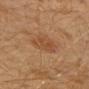biopsy_status: not biopsied; imaged during a skin examination
image:
  source: total-body photography crop
  field_of_view_mm: 15
site: left arm
lighting: cross-polarized
patient:
  sex: male
  age_approx: 30
lesion_size:
  long_diameter_mm_approx: 4.0
automated_metrics:
  area_mm2_approx: 8.5
  eccentricity: 0.6
  shape_asymmetry: 0.2
  nevus_likeness_0_100: 40
  lesion_detection_confidence_0_100: 100